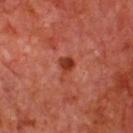Clinical summary:
This image is a 15 mm lesion crop taken from a total-body photograph. The lesion's longest dimension is about 3 mm. Imaged with cross-polarized lighting. Located on the chest. The patient is a male aged 63 to 67.